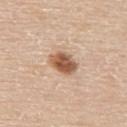notes: no biopsy performed (imaged during a skin exam) | automated lesion analysis: an area of roughly 7 mm², a shape eccentricity near 0.7, and a shape-asymmetry score of about 0.2 (0 = symmetric); a nevus-likeness score of about 95/100 and lesion-presence confidence of about 100/100 | image source: ~15 mm tile from a whole-body skin photo | patient: male, approximately 60 years of age | site: the upper back.